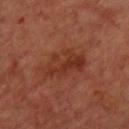follow-up: catalogued during a skin exam; not biopsied | size: about 5.5 mm | site: the upper back | subject: male, roughly 65 years of age | tile lighting: cross-polarized | imaging modality: ~15 mm crop, total-body skin-cancer survey.